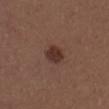This lesion was catalogued during total-body skin photography and was not selected for biopsy. The subject is a male aged approximately 30. An algorithmic analysis of the crop reported an average lesion color of about L≈33 a*≈19 b*≈23 (CIELAB), about 10 CIELAB-L* units darker than the surrounding skin, and a normalized lesion–skin contrast near 9. And it measured a border-irregularity index near 1.5/10 and a peripheral color-asymmetry measure near 1. And it measured an automated nevus-likeness rating near 85 out of 100 and lesion-presence confidence of about 100/100. The lesion is located on the right upper arm. Imaged with white-light lighting. Approximately 3 mm at its widest. Cropped from a total-body skin-imaging series; the visible field is about 15 mm.